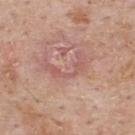Imaged during a routine full-body skin examination; the lesion was not biopsied and no histopathology is available. From the upper back. A male patient, roughly 60 years of age. The lesion's longest dimension is about 5 mm. Imaged with white-light lighting. This image is a 15 mm lesion crop taken from a total-body photograph.